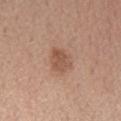biopsy status: total-body-photography surveillance lesion; no biopsy | automated metrics: a lesion area of about 6 mm² and two-axis asymmetry of about 0.25; a lesion color around L≈53 a*≈21 b*≈30 in CIELAB and about 9 CIELAB-L* units darker than the surrounding skin; a border-irregularity rating of about 2/10 and peripheral color asymmetry of about 1 | image source: 15 mm crop, total-body photography | lesion size: ≈3 mm | body site: the right forearm | patient: female, aged around 40 | lighting: white-light illumination.